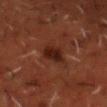<tbp_lesion>
  <biopsy_status>not biopsied; imaged during a skin examination</biopsy_status>
  <site>head or neck</site>
  <patient>
    <sex>male</sex>
    <age_approx>50</age_approx>
  </patient>
  <automated_metrics>
    <cielab_L>17</cielab_L>
    <cielab_a>19</cielab_a>
    <cielab_b>20</cielab_b>
    <vs_skin_darker_L>8.0</vs_skin_darker_L>
    <vs_skin_contrast_norm>10.0</vs_skin_contrast_norm>
    <color_variation_0_10>3.0</color_variation_0_10>
    <peripheral_color_asymmetry>1.0</peripheral_color_asymmetry>
    <nevus_likeness_0_100>75</nevus_likeness_0_100>
    <lesion_detection_confidence_0_100>100</lesion_detection_confidence_0_100>
  </automated_metrics>
  <lesion_size>
    <long_diameter_mm_approx>3.0</long_diameter_mm_approx>
  </lesion_size>
  <lighting>cross-polarized</lighting>
  <image>
    <source>total-body photography crop</source>
    <field_of_view_mm>15</field_of_view_mm>
  </image>
</tbp_lesion>